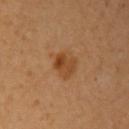follow-up: no biopsy performed (imaged during a skin exam)
image source: ~15 mm crop, total-body skin-cancer survey
tile lighting: cross-polarized illumination
patient: female, about 35 years old
anatomic site: the left upper arm
size: ~2.5 mm (longest diameter)
automated metrics: an eccentricity of roughly 0.8 and a shape-asymmetry score of about 0.2 (0 = symmetric); a mean CIELAB color near L≈42 a*≈23 b*≈37, roughly 10 lightness units darker than nearby skin, and a normalized lesion–skin contrast near 8; border irregularity of about 2.5 on a 0–10 scale, a within-lesion color-variation index near 4/10, and peripheral color asymmetry of about 1.5